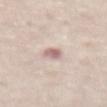| key | value |
|---|---|
| workup | catalogued during a skin exam; not biopsied |
| size | about 2.5 mm |
| subject | female, aged 68–72 |
| image source | ~15 mm crop, total-body skin-cancer survey |
| tile lighting | white-light illumination |
| site | the abdomen |